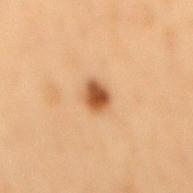biopsy_status: not biopsied; imaged during a skin examination
site: mid back
patient:
  sex: male
  age_approx: 55
image:
  source: total-body photography crop
  field_of_view_mm: 15
automated_metrics:
  area_mm2_approx: 4.5
  eccentricity: 0.65
  shape_asymmetry: 0.15
  border_irregularity_0_10: 1.5
  color_variation_0_10: 3.0
  peripheral_color_asymmetry: 1.0
  lesion_detection_confidence_0_100: 100